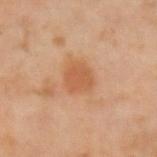follow-up: catalogued during a skin exam; not biopsied.